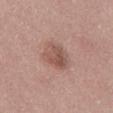The lesion was photographed on a routine skin check and not biopsied; there is no pathology result. The lesion is located on the lower back. A region of skin cropped from a whole-body photographic capture, roughly 15 mm wide. Imaged with white-light lighting. The patient is a male aged 28 to 32. The lesion's longest dimension is about 3.5 mm. The total-body-photography lesion software estimated a lesion-to-skin contrast of about 6.5 (normalized; higher = more distinct). The software also gave a border-irregularity rating of about 1.5/10, a within-lesion color-variation index near 5/10, and radial color variation of about 2. The analysis additionally found an automated nevus-likeness rating near 25 out of 100 and a lesion-detection confidence of about 100/100.